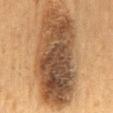{"biopsy_status": "not biopsied; imaged during a skin examination", "site": "mid back", "patient": {"sex": "male", "age_approx": 60}, "image": {"source": "total-body photography crop", "field_of_view_mm": 15}, "automated_metrics": {"area_mm2_approx": 60.0, "eccentricity": 0.9, "shape_asymmetry": 0.15}}